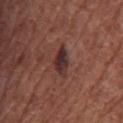Part of a total-body skin-imaging series; this lesion was reviewed on a skin check and was not flagged for biopsy. Cropped from a total-body skin-imaging series; the visible field is about 15 mm. Automated image analysis of the tile measured an area of roughly 6 mm², a shape eccentricity near 0.85, and a symmetry-axis asymmetry near 0.4. The analysis additionally found a mean CIELAB color near L≈32 a*≈20 b*≈20, a lesion–skin lightness drop of about 10, and a normalized border contrast of about 10.5. And it measured a detector confidence of about 100 out of 100 that the crop contains a lesion. The lesion is on the chest. A male subject, approximately 75 years of age.